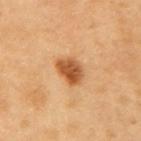Q: Was a biopsy performed?
A: total-body-photography surveillance lesion; no biopsy
Q: How was this image acquired?
A: ~15 mm tile from a whole-body skin photo
Q: Who is the patient?
A: male, aged 58 to 62
Q: Automated lesion metrics?
A: a mean CIELAB color near L≈54 a*≈25 b*≈41, roughly 15 lightness units darker than nearby skin, and a normalized lesion–skin contrast near 10; internal color variation of about 5 on a 0–10 scale and radial color variation of about 1.5; a nevus-likeness score of about 95/100 and a detector confidence of about 100 out of 100 that the crop contains a lesion
Q: What is the anatomic site?
A: the right upper arm
Q: What lighting was used for the tile?
A: cross-polarized illumination
Q: What is the lesion's diameter?
A: ~3.5 mm (longest diameter)